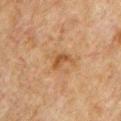Impression:
No biopsy was performed on this lesion — it was imaged during a full skin examination and was not determined to be concerning.
Acquisition and patient details:
Captured under cross-polarized illumination. Located on the chest. Automated image analysis of the tile measured a lesion area of about 3 mm², an eccentricity of roughly 0.85, and a symmetry-axis asymmetry near 0.4. It also reported a lesion color around L≈41 a*≈19 b*≈33 in CIELAB, a lesion–skin lightness drop of about 8, and a normalized border contrast of about 7. And it measured internal color variation of about 2 on a 0–10 scale. A male subject, aged 48–52. A region of skin cropped from a whole-body photographic capture, roughly 15 mm wide.